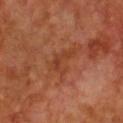  biopsy_status: not biopsied; imaged during a skin examination
  image:
    source: total-body photography crop
    field_of_view_mm: 15
  automated_metrics:
    cielab_L: 39
    cielab_a: 27
    cielab_b: 33
    vs_skin_darker_L: 6.0
    vs_skin_contrast_norm: 5.5
    border_irregularity_0_10: 7.5
    color_variation_0_10: 1.0
    peripheral_color_asymmetry: 0.5
  lesion_size:
    long_diameter_mm_approx: 3.5
  site: chest
  patient:
    sex: male
    age_approx: 65